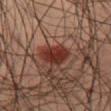No biopsy was performed on this lesion — it was imaged during a full skin examination and was not determined to be concerning.
The patient is a male aged around 50.
This is a cross-polarized tile.
From the left thigh.
A close-up tile cropped from a whole-body skin photograph, about 15 mm across.